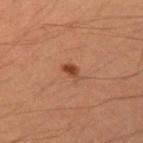Recorded during total-body skin imaging; not selected for excision or biopsy. Automated image analysis of the tile measured an average lesion color of about L≈44 a*≈26 b*≈34 (CIELAB) and a lesion–skin lightness drop of about 11. From the left upper arm. The lesion's longest dimension is about 2.5 mm. A male subject, aged 33 to 37. Cropped from a whole-body photographic skin survey; the tile spans about 15 mm. Captured under cross-polarized illumination.